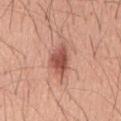follow-up: total-body-photography surveillance lesion; no biopsy
body site: the back
lesion size: ~4.5 mm (longest diameter)
patient: male, aged approximately 60
illumination: white-light illumination
acquisition: 15 mm crop, total-body photography